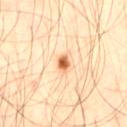The lesion was photographed on a routine skin check and not biopsied; there is no pathology result.